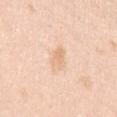Clinical impression:
Captured during whole-body skin photography for melanoma surveillance; the lesion was not biopsied.
Context:
Imaged with white-light lighting. The lesion is located on the arm. A close-up tile cropped from a whole-body skin photograph, about 15 mm across. A female patient, aged around 40. The lesion's longest dimension is about 3.5 mm.